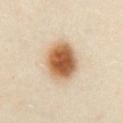Imaged during a routine full-body skin examination; the lesion was not biopsied and no histopathology is available.
A female subject, aged 38–42.
The lesion is located on the abdomen.
A region of skin cropped from a whole-body photographic capture, roughly 15 mm wide.